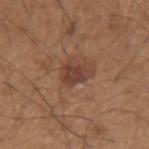{"biopsy_status": "not biopsied; imaged during a skin examination", "lighting": "white-light", "image": {"source": "total-body photography crop", "field_of_view_mm": 15}, "patient": {"sex": "male", "age_approx": 65}, "automated_metrics": {"lesion_detection_confidence_0_100": 100}, "site": "left upper arm", "lesion_size": {"long_diameter_mm_approx": 2.5}}